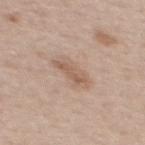Assessment: The lesion was tiled from a total-body skin photograph and was not biopsied. Acquisition and patient details: The lesion is located on the back. The lesion's longest dimension is about 4.5 mm. Cropped from a total-body skin-imaging series; the visible field is about 15 mm. This is a white-light tile. The subject is a female roughly 40 years of age.